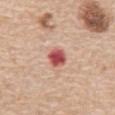Notes:
- image source — 15 mm crop, total-body photography
- location — the abdomen
- subject — male, about 70 years old
- lighting — white-light